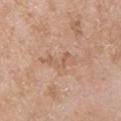notes: total-body-photography surveillance lesion; no biopsy
lesion size: ~4.5 mm (longest diameter)
acquisition: total-body-photography crop, ~15 mm field of view
lighting: white-light illumination
automated metrics: an average lesion color of about L≈59 a*≈19 b*≈32 (CIELAB), roughly 7 lightness units darker than nearby skin, and a normalized border contrast of about 5; a border-irregularity rating of about 8/10, a color-variation rating of about 0.5/10, and radial color variation of about 0.5
anatomic site: the left upper arm
patient: female, approximately 70 years of age